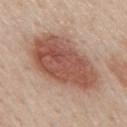follow-up: total-body-photography surveillance lesion; no biopsy | lesion diameter: ~9.5 mm (longest diameter) | anatomic site: the mid back | patient: male, aged 58 to 62 | lighting: white-light illumination | acquisition: total-body-photography crop, ~15 mm field of view.